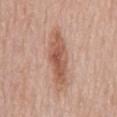Imaged during a routine full-body skin examination; the lesion was not biopsied and no histopathology is available. The subject is a male roughly 80 years of age. This is a white-light tile. An algorithmic analysis of the crop reported an eccentricity of roughly 0.95. The analysis additionally found an automated nevus-likeness rating near 70 out of 100 and lesion-presence confidence of about 100/100. On the mid back. Cropped from a whole-body photographic skin survey; the tile spans about 15 mm. Longest diameter approximately 6.5 mm.